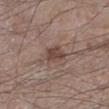Clinical impression:
Captured during whole-body skin photography for melanoma surveillance; the lesion was not biopsied.
Image and clinical context:
This image is a 15 mm lesion crop taken from a total-body photograph. Approximately 3 mm at its widest. A male subject aged around 55. Located on the leg. The tile uses white-light illumination.